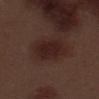The lesion was tiled from a total-body skin photograph and was not biopsied.
Measured at roughly 5 mm in maximum diameter.
From the leg.
A 15 mm close-up extracted from a 3D total-body photography capture.
Automated tile analysis of the lesion measured border irregularity of about 2 on a 0–10 scale and radial color variation of about 1.
A male patient, roughly 70 years of age.